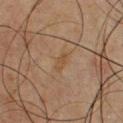Q: Was this lesion biopsied?
A: no biopsy performed (imaged during a skin exam)
Q: Where on the body is the lesion?
A: the chest
Q: Patient demographics?
A: male, about 45 years old
Q: Illumination type?
A: cross-polarized
Q: How was this image acquired?
A: ~15 mm crop, total-body skin-cancer survey
Q: Lesion size?
A: ~2.5 mm (longest diameter)